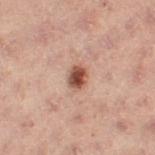workup: total-body-photography surveillance lesion; no biopsy
diameter: ~2.5 mm (longest diameter)
image source: 15 mm crop, total-body photography
body site: the left thigh
automated lesion analysis: a lesion area of about 4.5 mm² and an eccentricity of roughly 0.7; a lesion color around L≈42 a*≈20 b*≈24 in CIELAB, roughly 13 lightness units darker than nearby skin, and a lesion-to-skin contrast of about 10.5 (normalized; higher = more distinct); a border-irregularity index near 1.5/10 and radial color variation of about 1
subject: female, aged 53 to 57
lighting: cross-polarized illumination